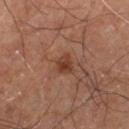Impression: No biopsy was performed on this lesion — it was imaged during a full skin examination and was not determined to be concerning. Clinical summary: Longest diameter approximately 2.5 mm. The lesion is located on the left thigh. The lesion-visualizer software estimated a footprint of about 3.5 mm², an eccentricity of roughly 0.6, and two-axis asymmetry of about 0.25. It also reported an average lesion color of about L≈35 a*≈22 b*≈27 (CIELAB), about 8 CIELAB-L* units darker than the surrounding skin, and a normalized lesion–skin contrast near 8. And it measured border irregularity of about 2 on a 0–10 scale, a within-lesion color-variation index near 4/10, and radial color variation of about 1.5. A male subject, aged 58 to 62. A lesion tile, about 15 mm wide, cut from a 3D total-body photograph.